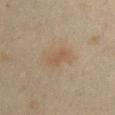tile lighting: cross-polarized illumination
diameter: ~3 mm (longest diameter)
image: 15 mm crop, total-body photography
subject: male, approximately 50 years of age
automated lesion analysis: a footprint of about 4 mm², a shape eccentricity near 0.9, and a shape-asymmetry score of about 0.3 (0 = symmetric)
location: the chest